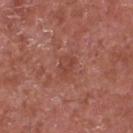Assessment: This lesion was catalogued during total-body skin photography and was not selected for biopsy. Context: A male subject approximately 65 years of age. Located on the chest. The tile uses white-light illumination. A lesion tile, about 15 mm wide, cut from a 3D total-body photograph. Approximately 3 mm at its widest.